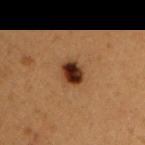Q: Is there a histopathology result?
A: imaged on a skin check; not biopsied
Q: How was this image acquired?
A: total-body-photography crop, ~15 mm field of view
Q: Patient demographics?
A: male, aged 48 to 52
Q: Where on the body is the lesion?
A: the arm
Q: Automated lesion metrics?
A: border irregularity of about 1 on a 0–10 scale, a color-variation rating of about 7/10, and peripheral color asymmetry of about 2
Q: What lighting was used for the tile?
A: cross-polarized